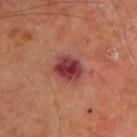Q: Was this lesion biopsied?
A: imaged on a skin check; not biopsied
Q: Illumination type?
A: cross-polarized
Q: Automated lesion metrics?
A: border irregularity of about 2 on a 0–10 scale and a peripheral color-asymmetry measure near 2.5; a nevus-likeness score of about 0/100 and a lesion-detection confidence of about 100/100
Q: What is the imaging modality?
A: ~15 mm crop, total-body skin-cancer survey
Q: Who is the patient?
A: male, approximately 65 years of age
Q: Lesion size?
A: ≈3.5 mm
Q: Where on the body is the lesion?
A: the upper back